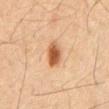workup: no biopsy performed (imaged during a skin exam) | size: about 3.5 mm | body site: the mid back | patient: male, roughly 65 years of age | acquisition: 15 mm crop, total-body photography | TBP lesion metrics: an area of roughly 6.5 mm², an eccentricity of roughly 0.75, and a symmetry-axis asymmetry near 0.2; an average lesion color of about L≈47 a*≈20 b*≈32 (CIELAB), a lesion–skin lightness drop of about 13, and a normalized border contrast of about 9.5; border irregularity of about 2 on a 0–10 scale and a peripheral color-asymmetry measure near 1.5; a nevus-likeness score of about 100/100 and a detector confidence of about 100 out of 100 that the crop contains a lesion | illumination: cross-polarized illumination.